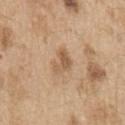Q: Was this lesion biopsied?
A: no biopsy performed (imaged during a skin exam)
Q: Lesion size?
A: about 3.5 mm
Q: What is the anatomic site?
A: the left upper arm
Q: Patient demographics?
A: male, in their 70s
Q: What is the imaging modality?
A: 15 mm crop, total-body photography
Q: Illumination type?
A: white-light illumination